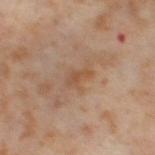biopsy status = catalogued during a skin exam; not biopsied | subject = female, roughly 55 years of age | lesion diameter = about 3 mm | tile lighting = cross-polarized illumination | location = the right thigh | acquisition = ~15 mm tile from a whole-body skin photo.